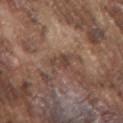{
  "biopsy_status": "not biopsied; imaged during a skin examination",
  "image": {
    "source": "total-body photography crop",
    "field_of_view_mm": 15
  },
  "lesion_size": {
    "long_diameter_mm_approx": 3.0
  },
  "site": "arm",
  "patient": {
    "sex": "male",
    "age_approx": 75
  },
  "lighting": "white-light"
}